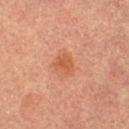No biopsy was performed on this lesion — it was imaged during a full skin examination and was not determined to be concerning.
A female patient about 60 years old.
The lesion-visualizer software estimated a footprint of about 5 mm², an outline eccentricity of about 0.3 (0 = round, 1 = elongated), and a shape-asymmetry score of about 0.25 (0 = symmetric).
The lesion is on the left thigh.
This image is a 15 mm lesion crop taken from a total-body photograph.